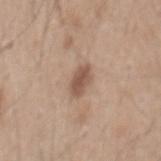The lesion was tiled from a total-body skin photograph and was not biopsied.
Located on the mid back.
Automated tile analysis of the lesion measured a footprint of about 5 mm², a shape eccentricity near 0.85, and two-axis asymmetry of about 0.2. The software also gave a mean CIELAB color near L≈53 a*≈18 b*≈27, a lesion–skin lightness drop of about 12, and a normalized border contrast of about 8. The software also gave a border-irregularity index near 2/10, internal color variation of about 2 on a 0–10 scale, and peripheral color asymmetry of about 0.5.
A close-up tile cropped from a whole-body skin photograph, about 15 mm across.
A male subject, aged 43 to 47.